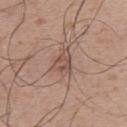<lesion>
<biopsy_status>not biopsied; imaged during a skin examination</biopsy_status>
<image>
  <source>total-body photography crop</source>
  <field_of_view_mm>15</field_of_view_mm>
</image>
<site>upper back</site>
<automated_metrics>
  <eccentricity>0.5</eccentricity>
  <shape_asymmetry>0.35</shape_asymmetry>
</automated_metrics>
<patient>
  <sex>male</sex>
  <age_approx>50</age_approx>
</patient>
<lighting>white-light</lighting>
</lesion>